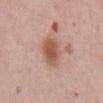Q: Was this lesion biopsied?
A: no biopsy performed (imaged during a skin exam)
Q: How was this image acquired?
A: total-body-photography crop, ~15 mm field of view
Q: Who is the patient?
A: female, in their mid- to late 60s
Q: What is the lesion's diameter?
A: ~4 mm (longest diameter)
Q: What is the anatomic site?
A: the abdomen